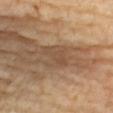The lesion was photographed on a routine skin check and not biopsied; there is no pathology result.